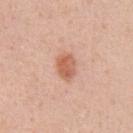{"biopsy_status": "not biopsied; imaged during a skin examination", "site": "abdomen", "patient": {"sex": "male", "age_approx": 55}, "image": {"source": "total-body photography crop", "field_of_view_mm": 15}}